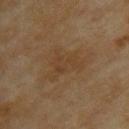Assessment:
The lesion was tiled from a total-body skin photograph and was not biopsied.
Context:
A female patient, aged approximately 60. An algorithmic analysis of the crop reported a lesion area of about 11 mm², a shape eccentricity near 0.65, and a shape-asymmetry score of about 0.5 (0 = symmetric). The recorded lesion diameter is about 5 mm. A region of skin cropped from a whole-body photographic capture, roughly 15 mm wide. From the upper back. This is a cross-polarized tile.